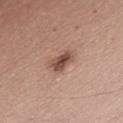workup — total-body-photography surveillance lesion; no biopsy | location — the abdomen | automated lesion analysis — a footprint of about 5 mm², a shape eccentricity near 0.85, and two-axis asymmetry of about 0.25; a border-irregularity rating of about 2.5/10 | image — ~15 mm crop, total-body skin-cancer survey | subject — male, approximately 55 years of age | tile lighting — white-light illumination | lesion diameter — ~3 mm (longest diameter).